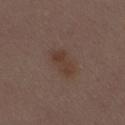  biopsy_status: not biopsied; imaged during a skin examination
  patient:
    sex: female
    age_approx: 30
  lighting: white-light
  site: mid back
  image:
    source: total-body photography crop
    field_of_view_mm: 15